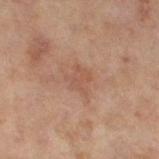Notes:
• workup · no biopsy performed (imaged during a skin exam)
• lighting · cross-polarized
• image source · total-body-photography crop, ~15 mm field of view
• patient · female, in their 60s
• site · the left thigh
• diameter · ~3 mm (longest diameter)